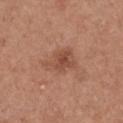This lesion was catalogued during total-body skin photography and was not selected for biopsy. A close-up tile cropped from a whole-body skin photograph, about 15 mm across. The recorded lesion diameter is about 4 mm. This is a white-light tile. The lesion is located on the chest. A female subject, aged approximately 40. The lesion-visualizer software estimated a color-variation rating of about 3.5/10. It also reported an automated nevus-likeness rating near 50 out of 100.